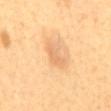Assessment: The lesion was tiled from a total-body skin photograph and was not biopsied. Context: Captured under cross-polarized illumination. Located on the abdomen. A 15 mm crop from a total-body photograph taken for skin-cancer surveillance. A male patient aged 48 to 52. Longest diameter approximately 3.5 mm.